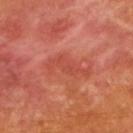The lesion was tiled from a total-body skin photograph and was not biopsied.
Automated tile analysis of the lesion measured a footprint of about 8 mm² and a shape-asymmetry score of about 0.45 (0 = symmetric). The analysis additionally found about 6 CIELAB-L* units darker than the surrounding skin and a normalized lesion–skin contrast near 4.5. The analysis additionally found an automated nevus-likeness rating near 0 out of 100 and lesion-presence confidence of about 100/100.
This is a cross-polarized tile.
Approximately 5 mm at its widest.
Cropped from a whole-body photographic skin survey; the tile spans about 15 mm.
The lesion is located on the back.
A female patient, aged approximately 55.